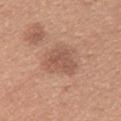{"biopsy_status": "not biopsied; imaged during a skin examination", "image": {"source": "total-body photography crop", "field_of_view_mm": 15}, "patient": {"sex": "female", "age_approx": 30}, "site": "back"}